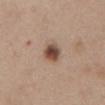The lesion was photographed on a routine skin check and not biopsied; there is no pathology result.
A female subject, aged around 40.
The lesion is located on the abdomen.
A 15 mm close-up extracted from a 3D total-body photography capture.
The lesion-visualizer software estimated a lesion area of about 5.5 mm², an outline eccentricity of about 0.55 (0 = round, 1 = elongated), and a symmetry-axis asymmetry near 0.2. The analysis additionally found a mean CIELAB color near L≈48 a*≈18 b*≈26. And it measured a classifier nevus-likeness of about 100/100.